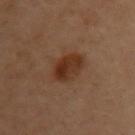Recorded during total-body skin imaging; not selected for excision or biopsy.
The lesion is located on the chest.
Automated image analysis of the tile measured an average lesion color of about L≈28 a*≈18 b*≈26 (CIELAB) and about 8 CIELAB-L* units darker than the surrounding skin. And it measured a color-variation rating of about 4.5/10. It also reported a nevus-likeness score of about 90/100 and a detector confidence of about 100 out of 100 that the crop contains a lesion.
Imaged with cross-polarized lighting.
A lesion tile, about 15 mm wide, cut from a 3D total-body photograph.
Longest diameter approximately 4.5 mm.
A female patient, in their 60s.